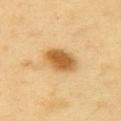Acquisition and patient details: Cropped from a whole-body photographic skin survey; the tile spans about 15 mm. The lesion's longest dimension is about 4.5 mm. From the left upper arm. A male subject aged 58 to 62. The tile uses cross-polarized illumination.